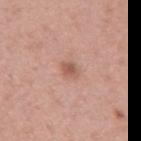Q: What kind of image is this?
A: 15 mm crop, total-body photography
Q: What are the patient's age and sex?
A: male, aged 38–42
Q: What is the lesion's diameter?
A: ~2.5 mm (longest diameter)
Q: How was the tile lit?
A: white-light illumination
Q: Lesion location?
A: the mid back